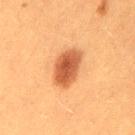• follow-up — imaged on a skin check; not biopsied
• anatomic site — the left thigh
• image-analysis metrics — an area of roughly 11 mm²; a mean CIELAB color near L≈47 a*≈25 b*≈36, about 14 CIELAB-L* units darker than the surrounding skin, and a normalized lesion–skin contrast near 10; a border-irregularity rating of about 1.5/10 and internal color variation of about 3.5 on a 0–10 scale; an automated nevus-likeness rating near 100 out of 100
• subject — female, roughly 40 years of age
• tile lighting — cross-polarized illumination
• image source — ~15 mm tile from a whole-body skin photo
• diameter — ≈5 mm